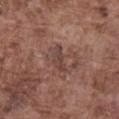- workup · imaged on a skin check; not biopsied
- automated lesion analysis · a lesion area of about 4.5 mm², a shape eccentricity near 0.9, and two-axis asymmetry of about 0.4
- anatomic site · the abdomen
- subject · male, about 75 years old
- lighting · white-light illumination
- image · 15 mm crop, total-body photography